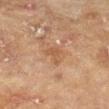Imaged during a routine full-body skin examination; the lesion was not biopsied and no histopathology is available. Automated tile analysis of the lesion measured a shape eccentricity near 0.85. It also reported internal color variation of about 0.5 on a 0–10 scale and peripheral color asymmetry of about 0. Cropped from a total-body skin-imaging series; the visible field is about 15 mm. A female subject, in their 80s. The lesion's longest dimension is about 3 mm. Located on the left forearm.